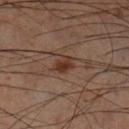Longest diameter approximately 3 mm.
An algorithmic analysis of the crop reported an eccentricity of roughly 0.75 and a shape-asymmetry score of about 0.2 (0 = symmetric). It also reported an average lesion color of about L≈33 a*≈18 b*≈25 (CIELAB), a lesion–skin lightness drop of about 9, and a lesion-to-skin contrast of about 8.5 (normalized; higher = more distinct).
A region of skin cropped from a whole-body photographic capture, roughly 15 mm wide.
The lesion is located on the left lower leg.
The subject is a male aged approximately 60.
The tile uses cross-polarized illumination.